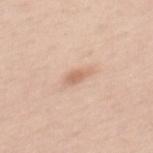follow-up — no biopsy performed (imaged during a skin exam) | subject — female, in their mid-30s | lighting — white-light | imaging modality — total-body-photography crop, ~15 mm field of view | automated lesion analysis — a lesion area of about 3 mm², an outline eccentricity of about 0.85 (0 = round, 1 = elongated), and a shape-asymmetry score of about 0.35 (0 = symmetric) | location — the mid back.